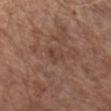Q: Is there a histopathology result?
A: catalogued during a skin exam; not biopsied
Q: What is the anatomic site?
A: the chest
Q: What did automated image analysis measure?
A: a footprint of about 4.5 mm² and an outline eccentricity of about 0.65 (0 = round, 1 = elongated); border irregularity of about 2 on a 0–10 scale; an automated nevus-likeness rating near 0 out of 100 and a detector confidence of about 100 out of 100 that the crop contains a lesion
Q: What is the lesion's diameter?
A: ~2.5 mm (longest diameter)
Q: What is the imaging modality?
A: ~15 mm tile from a whole-body skin photo
Q: What lighting was used for the tile?
A: white-light illumination
Q: Who is the patient?
A: male, aged approximately 80